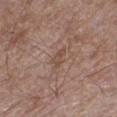follow-up: imaged on a skin check; not biopsied
subject: male, roughly 70 years of age
anatomic site: the left thigh
image source: ~15 mm tile from a whole-body skin photo